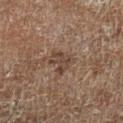{
  "biopsy_status": "not biopsied; imaged during a skin examination",
  "image": {
    "source": "total-body photography crop",
    "field_of_view_mm": 15
  },
  "site": "leg",
  "lesion_size": {
    "long_diameter_mm_approx": 3.0
  },
  "lighting": "cross-polarized",
  "automated_metrics": {
    "area_mm2_approx": 4.5,
    "eccentricity": 0.75,
    "shape_asymmetry": 0.5,
    "cielab_L": 30,
    "cielab_a": 13,
    "cielab_b": 20,
    "vs_skin_darker_L": 7.0,
    "vs_skin_contrast_norm": 7.0,
    "border_irregularity_0_10": 6.5,
    "color_variation_0_10": 1.5,
    "peripheral_color_asymmetry": 0.5
  },
  "patient": {
    "sex": "male",
    "age_approx": 60
  }
}